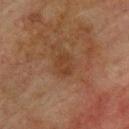Clinical impression:
Part of a total-body skin-imaging series; this lesion was reviewed on a skin check and was not flagged for biopsy.
Context:
The lesion's longest dimension is about 2.5 mm. A male subject approximately 75 years of age. The lesion is on the upper back. Imaged with cross-polarized lighting. A 15 mm crop from a total-body photograph taken for skin-cancer surveillance. Automated image analysis of the tile measured a color-variation rating of about 2/10 and a peripheral color-asymmetry measure near 0.5. The software also gave an automated nevus-likeness rating near 0 out of 100.